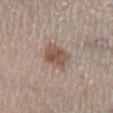Impression:
No biopsy was performed on this lesion — it was imaged during a full skin examination and was not determined to be concerning.
Context:
Imaged with white-light lighting. A close-up tile cropped from a whole-body skin photograph, about 15 mm across. The patient is a male aged around 60. On the left lower leg.